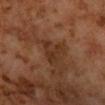biopsy status=total-body-photography surveillance lesion; no biopsy | patient=male, aged approximately 60 | site=the arm | imaging modality=~15 mm crop, total-body skin-cancer survey | illumination=cross-polarized.